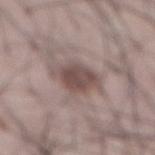workup: no biopsy performed (imaged during a skin exam) | site: the abdomen | subject: male, approximately 55 years of age | image source: 15 mm crop, total-body photography | lesion diameter: about 4 mm | tile lighting: white-light illumination.